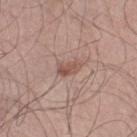Assessment: Captured during whole-body skin photography for melanoma surveillance; the lesion was not biopsied. Clinical summary: A male subject aged approximately 55. The tile uses white-light illumination. A close-up tile cropped from a whole-body skin photograph, about 15 mm across. An algorithmic analysis of the crop reported an average lesion color of about L≈52 a*≈20 b*≈25 (CIELAB), a lesion–skin lightness drop of about 10, and a normalized border contrast of about 7. The software also gave a classifier nevus-likeness of about 25/100 and lesion-presence confidence of about 100/100. Measured at roughly 2.5 mm in maximum diameter. The lesion is on the right lower leg.